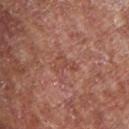Assessment: Recorded during total-body skin imaging; not selected for excision or biopsy. Context: A 15 mm close-up extracted from a 3D total-body photography capture. A male patient, in their mid-50s. The lesion is on the chest.